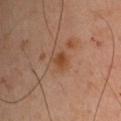No biopsy was performed on this lesion — it was imaged during a full skin examination and was not determined to be concerning. Automated tile analysis of the lesion measured a footprint of about 3 mm² and a symmetry-axis asymmetry near 0.25. The analysis additionally found an average lesion color of about L≈41 a*≈21 b*≈32 (CIELAB), roughly 8 lightness units darker than nearby skin, and a normalized border contrast of about 8. The software also gave a classifier nevus-likeness of about 20/100 and lesion-presence confidence of about 100/100. A male patient roughly 45 years of age. The lesion is on the left upper arm. About 2 mm across. A 15 mm close-up tile from a total-body photography series done for melanoma screening.